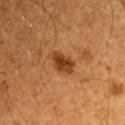notes: no biopsy performed (imaged during a skin exam)
acquisition: 15 mm crop, total-body photography
patient: male, aged 58 to 62
location: the left upper arm